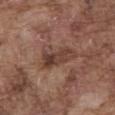Imaged during a routine full-body skin examination; the lesion was not biopsied and no histopathology is available.
Automated image analysis of the tile measured a lesion color around L≈40 a*≈19 b*≈25 in CIELAB and a lesion-to-skin contrast of about 8 (normalized; higher = more distinct). The analysis additionally found internal color variation of about 6.5 on a 0–10 scale and radial color variation of about 2.5.
A male subject, aged approximately 75.
About 5 mm across.
The lesion is on the abdomen.
This is a white-light tile.
Cropped from a whole-body photographic skin survey; the tile spans about 15 mm.